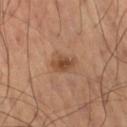biopsy status: no biopsy performed (imaged during a skin exam)
size: ≈3 mm
imaging modality: ~15 mm tile from a whole-body skin photo
patient: male, aged 68–72
tile lighting: cross-polarized
body site: the right thigh